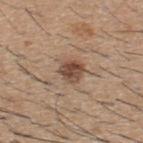follow-up: catalogued during a skin exam; not biopsied | acquisition: 15 mm crop, total-body photography | body site: the back | tile lighting: white-light illumination | patient: male, approximately 60 years of age | diameter: ≈3 mm | automated metrics: an area of roughly 6 mm², an eccentricity of roughly 0.25, and a shape-asymmetry score of about 0.2 (0 = symmetric).